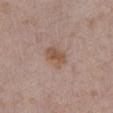Clinical impression: This lesion was catalogued during total-body skin photography and was not selected for biopsy. Clinical summary: A male patient about 55 years old. On the abdomen. A lesion tile, about 15 mm wide, cut from a 3D total-body photograph. The lesion's longest dimension is about 3 mm.